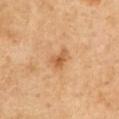follow-up=imaged on a skin check; not biopsied | illumination=cross-polarized illumination | imaging modality=15 mm crop, total-body photography | TBP lesion metrics=an eccentricity of roughly 0.75 and a symmetry-axis asymmetry near 0.4; an average lesion color of about L≈58 a*≈22 b*≈40 (CIELAB); border irregularity of about 3.5 on a 0–10 scale, internal color variation of about 3.5 on a 0–10 scale, and radial color variation of about 1 | size=~3 mm (longest diameter) | subject=male, aged 48–52 | site=the chest.